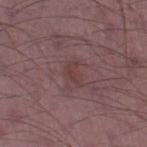| field | value |
|---|---|
| notes | total-body-photography surveillance lesion; no biopsy |
| illumination | white-light illumination |
| imaging modality | total-body-photography crop, ~15 mm field of view |
| patient | male, roughly 50 years of age |
| anatomic site | the left thigh |